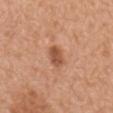Clinical impression:
Imaged during a routine full-body skin examination; the lesion was not biopsied and no histopathology is available.
Background:
The subject is a male roughly 70 years of age. A lesion tile, about 15 mm wide, cut from a 3D total-body photograph. From the mid back. The lesion's longest dimension is about 3 mm. Imaged with white-light lighting.